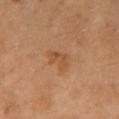Assessment: This lesion was catalogued during total-body skin photography and was not selected for biopsy. Image and clinical context: From the right upper arm. The subject is a female aged 68 to 72. Captured under cross-polarized illumination. This image is a 15 mm lesion crop taken from a total-body photograph.